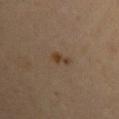The lesion is located on the left upper arm. Automated tile analysis of the lesion measured border irregularity of about 4 on a 0–10 scale, a within-lesion color-variation index near 1/10, and radial color variation of about 0.5. This is a cross-polarized tile. A female subject, aged 28 to 32. A roughly 15 mm field-of-view crop from a total-body skin photograph.